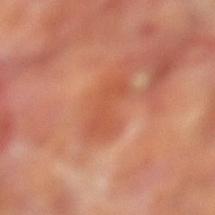Clinical summary:
A close-up tile cropped from a whole-body skin photograph, about 15 mm across. An algorithmic analysis of the crop reported an area of roughly 7 mm² and an outline eccentricity of about 0.9 (0 = round, 1 = elongated). The software also gave a mean CIELAB color near L≈52 a*≈30 b*≈36, a lesion–skin lightness drop of about 7, and a normalized border contrast of about 5. And it measured peripheral color asymmetry of about 0.5. Located on the leg. Captured under cross-polarized illumination. A male subject, aged around 70. The recorded lesion diameter is about 5 mm.